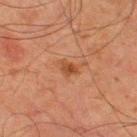No biopsy was performed on this lesion — it was imaged during a full skin examination and was not determined to be concerning.
Measured at roughly 2.5 mm in maximum diameter.
Automated image analysis of the tile measured a color-variation rating of about 3/10 and a peripheral color-asymmetry measure near 1.
Located on the right thigh.
A lesion tile, about 15 mm wide, cut from a 3D total-body photograph.
A male patient, in their 80s.